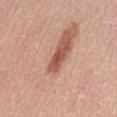biopsy status: catalogued during a skin exam; not biopsied | subject: male, aged approximately 50 | imaging modality: ~15 mm tile from a whole-body skin photo | size: about 2 mm | image-analysis metrics: a shape eccentricity near 0.9 and two-axis asymmetry of about 0.4; a border-irregularity index near 3.5/10 and a peripheral color-asymmetry measure near 0; a classifier nevus-likeness of about 0/100 and a lesion-detection confidence of about 30/100 | tile lighting: white-light illumination | anatomic site: the abdomen.